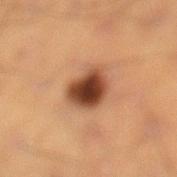<tbp_lesion>
<biopsy_status>not biopsied; imaged during a skin examination</biopsy_status>
<site>right lower leg</site>
<lighting>cross-polarized</lighting>
<automated_metrics>
  <cielab_L>39</cielab_L>
  <cielab_a>21</cielab_a>
  <cielab_b>29</cielab_b>
  <vs_skin_darker_L>16.0</vs_skin_darker_L>
</automated_metrics>
<image>
  <source>total-body photography crop</source>
  <field_of_view_mm>15</field_of_view_mm>
</image>
<patient>
  <sex>male</sex>
  <age_approx>55</age_approx>
</patient>
</tbp_lesion>